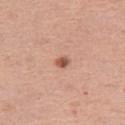biopsy status = catalogued during a skin exam; not biopsied | tile lighting = white-light | subject = female, about 55 years old | image = ~15 mm tile from a whole-body skin photo | anatomic site = the left thigh | lesion diameter = about 1.5 mm | image-analysis metrics = an eccentricity of roughly 0.55.